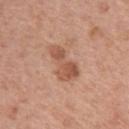<case>
  <biopsy_status>not biopsied; imaged during a skin examination</biopsy_status>
  <lighting>white-light</lighting>
  <site>left upper arm</site>
  <automated_metrics>
    <area_mm2_approx>9.0</area_mm2_approx>
    <eccentricity>0.85</eccentricity>
    <shape_asymmetry>0.45</shape_asymmetry>
  </automated_metrics>
  <patient>
    <sex>female</sex>
    <age_approx>40</age_approx>
  </patient>
  <lesion_size>
    <long_diameter_mm_approx>4.5</long_diameter_mm_approx>
  </lesion_size>
  <image>
    <source>total-body photography crop</source>
    <field_of_view_mm>15</field_of_view_mm>
  </image>
</case>